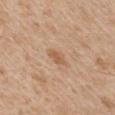The lesion was photographed on a routine skin check and not biopsied; there is no pathology result. This is a white-light tile. The lesion-visualizer software estimated a lesion color around L≈58 a*≈19 b*≈34 in CIELAB, roughly 8 lightness units darker than nearby skin, and a lesion-to-skin contrast of about 6 (normalized; higher = more distinct). And it measured a detector confidence of about 100 out of 100 that the crop contains a lesion. Approximately 2.5 mm at its widest. On the front of the torso. A male patient aged 63 to 67. This image is a 15 mm lesion crop taken from a total-body photograph.